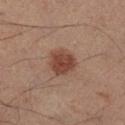Q: Is there a histopathology result?
A: total-body-photography surveillance lesion; no biopsy
Q: Lesion size?
A: about 3.5 mm
Q: What is the anatomic site?
A: the right lower leg
Q: What lighting was used for the tile?
A: cross-polarized illumination
Q: What kind of image is this?
A: ~15 mm tile from a whole-body skin photo
Q: Who is the patient?
A: male, aged approximately 50